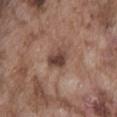{
  "biopsy_status": "not biopsied; imaged during a skin examination",
  "image": {
    "source": "total-body photography crop",
    "field_of_view_mm": 15
  },
  "site": "abdomen",
  "automated_metrics": {
    "cielab_L": 42,
    "cielab_a": 19,
    "cielab_b": 23,
    "vs_skin_darker_L": 12.0,
    "vs_skin_contrast_norm": 9.5,
    "border_irregularity_0_10": 2.5,
    "color_variation_0_10": 4.5,
    "peripheral_color_asymmetry": 1.5,
    "nevus_likeness_0_100": 45,
    "lesion_detection_confidence_0_100": 100
  },
  "lesion_size": {
    "long_diameter_mm_approx": 3.0
  },
  "patient": {
    "sex": "male",
    "age_approx": 75
  },
  "lighting": "white-light"
}